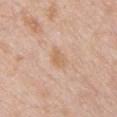  biopsy_status: not biopsied; imaged during a skin examination
  lesion_size:
    long_diameter_mm_approx: 3.0
  site: chest
  automated_metrics:
    border_irregularity_0_10: 2.0
    color_variation_0_10: 1.5
    lesion_detection_confidence_0_100: 100
  patient:
    sex: male
    age_approx: 50
  lighting: white-light
  image:
    source: total-body photography crop
    field_of_view_mm: 15